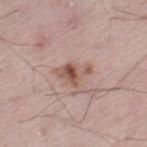Part of a total-body skin-imaging series; this lesion was reviewed on a skin check and was not flagged for biopsy. A male subject, aged 53 to 57. Located on the left thigh. A 15 mm close-up tile from a total-body photography series done for melanoma screening.